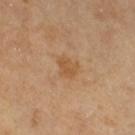<lesion>
  <biopsy_status>not biopsied; imaged during a skin examination</biopsy_status>
  <image>
    <source>total-body photography crop</source>
    <field_of_view_mm>15</field_of_view_mm>
  </image>
  <automated_metrics>
    <nevus_likeness_0_100>0</nevus_likeness_0_100>
  </automated_metrics>
  <lighting>cross-polarized</lighting>
  <site>leg</site>
  <patient>
    <sex>female</sex>
    <age_approx>55</age_approx>
  </patient>
</lesion>